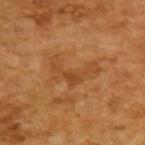About 5.5 mm across. A male patient in their mid- to late 60s. A 15 mm crop from a total-body photograph taken for skin-cancer surveillance. The tile uses cross-polarized illumination.